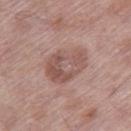Part of a total-body skin-imaging series; this lesion was reviewed on a skin check and was not flagged for biopsy. Automated image analysis of the tile measured a lesion color around L≈53 a*≈19 b*≈23 in CIELAB, roughly 9 lightness units darker than nearby skin, and a normalized border contrast of about 6.5. And it measured a lesion-detection confidence of about 100/100. A male patient aged 53 to 57. Measured at roughly 5 mm in maximum diameter. Located on the right thigh. A lesion tile, about 15 mm wide, cut from a 3D total-body photograph.